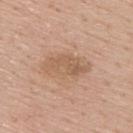Acquisition and patient details:
A lesion tile, about 15 mm wide, cut from a 3D total-body photograph. Located on the back. About 5.5 mm across. This is a white-light tile. A male patient, in their mid-50s.